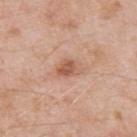Notes:
– workup — total-body-photography surveillance lesion; no biopsy
– subject — male, aged around 55
– size — ~3 mm (longest diameter)
– acquisition — total-body-photography crop, ~15 mm field of view
– tile lighting — white-light
– anatomic site — the left upper arm